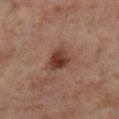Q: Was this lesion biopsied?
A: total-body-photography surveillance lesion; no biopsy